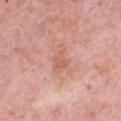{"biopsy_status": "not biopsied; imaged during a skin examination", "lesion_size": {"long_diameter_mm_approx": 3.5}, "patient": {"sex": "female", "age_approx": 65}, "lighting": "white-light", "site": "chest", "image": {"source": "total-body photography crop", "field_of_view_mm": 15}}